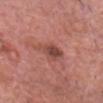About 3.5 mm across.
This is a white-light tile.
A 15 mm close-up tile from a total-body photography series done for melanoma screening.
The total-body-photography lesion software estimated an average lesion color of about L≈47 a*≈26 b*≈26 (CIELAB) and roughly 10 lightness units darker than nearby skin. The analysis additionally found a border-irregularity index near 3/10. And it measured an automated nevus-likeness rating near 10 out of 100.
On the head or neck.
The patient is a male in their 80s.